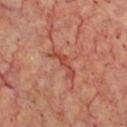Case summary:
– workup · catalogued during a skin exam; not biopsied
– image-analysis metrics · a border-irregularity index near 6.5/10, a color-variation rating of about 1.5/10, and peripheral color asymmetry of about 0
– patient · about 65 years old
– image source · ~15 mm tile from a whole-body skin photo
– lighting · cross-polarized
– body site · the front of the torso